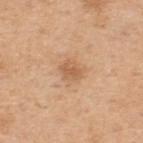<lesion>
  <biopsy_status>not biopsied; imaged during a skin examination</biopsy_status>
  <lighting>white-light</lighting>
  <site>upper back</site>
  <patient>
    <sex>male</sex>
    <age_approx>50</age_approx>
  </patient>
  <image>
    <source>total-body photography crop</source>
    <field_of_view_mm>15</field_of_view_mm>
  </image>
</lesion>